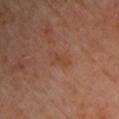| key | value |
|---|---|
| workup | catalogued during a skin exam; not biopsied |
| automated lesion analysis | a footprint of about 3 mm², an eccentricity of roughly 0.9, and two-axis asymmetry of about 0.45; roughly 5 lightness units darker than nearby skin and a lesion-to-skin contrast of about 5 (normalized; higher = more distinct) |
| image | 15 mm crop, total-body photography |
| anatomic site | the chest |
| lesion size | ≈3 mm |
| subject | male, in their mid-60s |